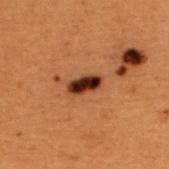The lesion was tiled from a total-body skin photograph and was not biopsied.
The patient is a male roughly 50 years of age.
Approximately 3.5 mm at its widest.
The lesion is located on the back.
A 15 mm crop from a total-body photograph taken for skin-cancer surveillance.
The tile uses cross-polarized illumination.